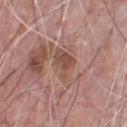Recorded during total-body skin imaging; not selected for excision or biopsy.
The lesion is located on the chest.
Automated image analysis of the tile measured a lesion area of about 6.5 mm² and an outline eccentricity of about 0.8 (0 = round, 1 = elongated). And it measured a lesion color around L≈50 a*≈19 b*≈27 in CIELAB, roughly 8 lightness units darker than nearby skin, and a normalized border contrast of about 7. The software also gave border irregularity of about 4.5 on a 0–10 scale and internal color variation of about 3 on a 0–10 scale. The software also gave an automated nevus-likeness rating near 0 out of 100.
This image is a 15 mm lesion crop taken from a total-body photograph.
Approximately 4 mm at its widest.
A male subject about 70 years old.